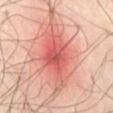| key | value |
|---|---|
| workup | imaged on a skin check; not biopsied |
| lesion diameter | about 8 mm |
| body site | the abdomen |
| patient | male, in their mid-40s |
| automated metrics | a lesion area of about 26 mm², an outline eccentricity of about 0.8 (0 = round, 1 = elongated), and a symmetry-axis asymmetry near 0.2; a classifier nevus-likeness of about 5/100 |
| image | ~15 mm crop, total-body skin-cancer survey |
| tile lighting | cross-polarized |